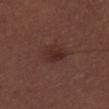The lesion was photographed on a routine skin check and not biopsied; there is no pathology result. Imaged with white-light lighting. Cropped from a total-body skin-imaging series; the visible field is about 15 mm. Automated tile analysis of the lesion measured a peripheral color-asymmetry measure near 1. And it measured an automated nevus-likeness rating near 75 out of 100 and a lesion-detection confidence of about 100/100. A male subject, aged 28–32. The lesion is on the upper back.